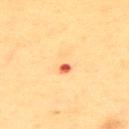Case summary:
- acquisition · 15 mm crop, total-body photography
- location · the upper back
- subject · female, in their mid-50s
- lesion diameter · about 3.5 mm